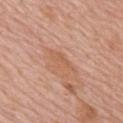| key | value |
|---|---|
| notes | no biopsy performed (imaged during a skin exam) |